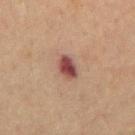Q: Was this lesion biopsied?
A: imaged on a skin check; not biopsied
Q: Lesion location?
A: the mid back
Q: How was this image acquired?
A: total-body-photography crop, ~15 mm field of view
Q: What are the patient's age and sex?
A: male, aged approximately 70
Q: Automated lesion metrics?
A: a lesion color around L≈38 a*≈20 b*≈19 in CIELAB, roughly 13 lightness units darker than nearby skin, and a normalized border contrast of about 11.5; a nevus-likeness score of about 0/100 and a detector confidence of about 100 out of 100 that the crop contains a lesion